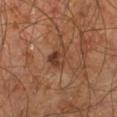Captured during whole-body skin photography for melanoma surveillance; the lesion was not biopsied.
About 3 mm across.
The lesion is on the right leg.
A male subject in their 60s.
A roughly 15 mm field-of-view crop from a total-body skin photograph.
The lesion-visualizer software estimated a shape eccentricity near 0.65 and two-axis asymmetry of about 0.35.